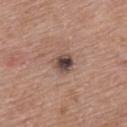Recorded during total-body skin imaging; not selected for excision or biopsy.
Measured at roughly 2.5 mm in maximum diameter.
The patient is a female approximately 50 years of age.
From the upper back.
A 15 mm close-up extracted from a 3D total-body photography capture.
The tile uses white-light illumination.